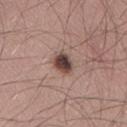Impression:
The lesion was tiled from a total-body skin photograph and was not biopsied.
Clinical summary:
The lesion is on the lower back. Longest diameter approximately 2.5 mm. A male patient in their mid-30s. This image is a 15 mm lesion crop taken from a total-body photograph.